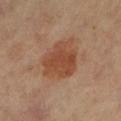Findings:
- anatomic site: the right leg
- lighting: cross-polarized
- automated metrics: a lesion area of about 16 mm², an eccentricity of roughly 0.55, and a symmetry-axis asymmetry near 0.25; a lesion color around L≈45 a*≈22 b*≈31 in CIELAB, a lesion–skin lightness drop of about 9, and a normalized lesion–skin contrast near 7.5; a border-irregularity rating of about 3/10, internal color variation of about 3.5 on a 0–10 scale, and a peripheral color-asymmetry measure near 1
- lesion size: about 5.5 mm
- patient: female, in their mid- to late 60s
- image source: total-body-photography crop, ~15 mm field of view